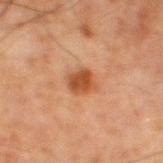Part of a total-body skin-imaging series; this lesion was reviewed on a skin check and was not flagged for biopsy.
Captured under cross-polarized illumination.
Automated tile analysis of the lesion measured a lesion color around L≈40 a*≈22 b*≈32 in CIELAB and a lesion-to-skin contrast of about 8.5 (normalized; higher = more distinct).
On the left thigh.
A region of skin cropped from a whole-body photographic capture, roughly 15 mm wide.
A male subject, aged 78–82.
About 3 mm across.